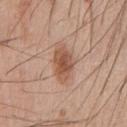Impression:
The lesion was tiled from a total-body skin photograph and was not biopsied.
Context:
The subject is a male aged 58–62. A 15 mm crop from a total-body photograph taken for skin-cancer surveillance. The lesion is on the chest.